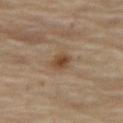Impression:
Imaged during a routine full-body skin examination; the lesion was not biopsied and no histopathology is available.
Acquisition and patient details:
On the right thigh. The subject is a female roughly 70 years of age. A roughly 15 mm field-of-view crop from a total-body skin photograph. The lesion-visualizer software estimated a footprint of about 4.5 mm², a shape eccentricity near 0.6, and a shape-asymmetry score of about 0.2 (0 = symmetric). It also reported an average lesion color of about L≈47 a*≈18 b*≈32 (CIELAB), about 10 CIELAB-L* units darker than the surrounding skin, and a lesion-to-skin contrast of about 8.5 (normalized; higher = more distinct).